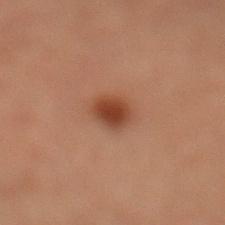The lesion was photographed on a routine skin check and not biopsied; there is no pathology result.
A male subject aged 58 to 62.
Located on the left lower leg.
This is a cross-polarized tile.
Cropped from a total-body skin-imaging series; the visible field is about 15 mm.
The lesion's longest dimension is about 2.5 mm.